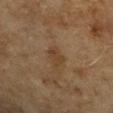Q: Was a biopsy performed?
A: catalogued during a skin exam; not biopsied
Q: What is the lesion's diameter?
A: about 2.5 mm
Q: What are the patient's age and sex?
A: female, about 60 years old
Q: Automated lesion metrics?
A: a border-irregularity index near 4/10, internal color variation of about 1.5 on a 0–10 scale, and peripheral color asymmetry of about 0.5; a nevus-likeness score of about 0/100 and a lesion-detection confidence of about 100/100
Q: Where on the body is the lesion?
A: the left forearm
Q: What kind of image is this?
A: ~15 mm tile from a whole-body skin photo